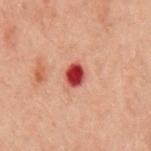Clinical impression:
Part of a total-body skin-imaging series; this lesion was reviewed on a skin check and was not flagged for biopsy.
Clinical summary:
From the chest. Longest diameter approximately 2.5 mm. A 15 mm crop from a total-body photograph taken for skin-cancer surveillance. A male subject, roughly 60 years of age. Imaged with cross-polarized lighting.